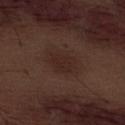No biopsy was performed on this lesion — it was imaged during a full skin examination and was not determined to be concerning. A region of skin cropped from a whole-body photographic capture, roughly 15 mm wide. The lesion-visualizer software estimated a lesion area of about 7 mm² and two-axis asymmetry of about 0.25. The analysis additionally found an average lesion color of about L≈24 a*≈17 b*≈20 (CIELAB) and a lesion–skin lightness drop of about 5. The analysis additionally found an automated nevus-likeness rating near 10 out of 100. Measured at roughly 3.5 mm in maximum diameter. On the abdomen. The subject is a male roughly 70 years of age. Captured under white-light illumination.